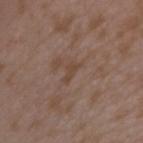Captured during whole-body skin photography for melanoma surveillance; the lesion was not biopsied.
The lesion is on the left upper arm.
About 2.5 mm across.
A lesion tile, about 15 mm wide, cut from a 3D total-body photograph.
The total-body-photography lesion software estimated a lesion area of about 2.5 mm² and a shape-asymmetry score of about 0.5 (0 = symmetric). And it measured border irregularity of about 5 on a 0–10 scale, internal color variation of about 0 on a 0–10 scale, and peripheral color asymmetry of about 0. And it measured an automated nevus-likeness rating near 0 out of 100 and lesion-presence confidence of about 100/100.
A female subject, approximately 35 years of age.